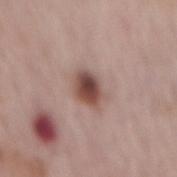Q: Patient demographics?
A: male, aged around 65
Q: What is the lesion's diameter?
A: about 3.5 mm
Q: Where on the body is the lesion?
A: the mid back
Q: What is the imaging modality?
A: ~15 mm tile from a whole-body skin photo